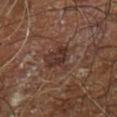{"biopsy_status": "not biopsied; imaged during a skin examination", "image": {"source": "total-body photography crop", "field_of_view_mm": 15}, "site": "leg", "automated_metrics": {"eccentricity": 0.7, "nevus_likeness_0_100": 0, "lesion_detection_confidence_0_100": 85}, "lighting": "cross-polarized", "patient": {"sex": "male", "age_approx": 60}}